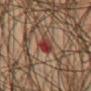Clinical impression: Part of a total-body skin-imaging series; this lesion was reviewed on a skin check and was not flagged for biopsy. Acquisition and patient details: A roughly 15 mm field-of-view crop from a total-body skin photograph. Imaged with cross-polarized lighting. A male subject aged approximately 75. The lesion is located on the chest.